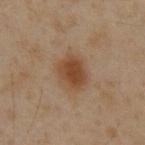Q: Was this lesion biopsied?
A: total-body-photography surveillance lesion; no biopsy
Q: What lighting was used for the tile?
A: cross-polarized
Q: What are the patient's age and sex?
A: male, aged around 60
Q: What is the lesion's diameter?
A: ≈4 mm
Q: What did automated image analysis measure?
A: an area of roughly 9.5 mm², an outline eccentricity of about 0.6 (0 = round, 1 = elongated), and two-axis asymmetry of about 0.2; an average lesion color of about L≈40 a*≈17 b*≈30 (CIELAB) and roughly 9 lightness units darker than nearby skin
Q: What is the imaging modality?
A: ~15 mm crop, total-body skin-cancer survey
Q: Where on the body is the lesion?
A: the front of the torso